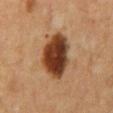Case summary:
• biopsy status — no biopsy performed (imaged during a skin exam)
• diameter — about 5.5 mm
• subject — male, aged 58 to 62
• image-analysis metrics — a within-lesion color-variation index near 6/10 and a peripheral color-asymmetry measure near 2; a nevus-likeness score of about 100/100 and a detector confidence of about 100 out of 100 that the crop contains a lesion
• site — the mid back
• acquisition — ~15 mm tile from a whole-body skin photo
• tile lighting — cross-polarized illumination